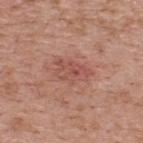subject = male, about 40 years old; automated metrics = an average lesion color of about L≈52 a*≈25 b*≈27 (CIELAB), a lesion–skin lightness drop of about 8, and a normalized border contrast of about 5.5; location = the upper back; lighting = white-light illumination; lesion diameter = ~4.5 mm (longest diameter); image = 15 mm crop, total-body photography.